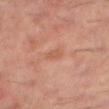Q: Is there a histopathology result?
A: catalogued during a skin exam; not biopsied
Q: What is the imaging modality?
A: 15 mm crop, total-body photography
Q: Lesion location?
A: the right thigh
Q: What are the patient's age and sex?
A: male, about 60 years old